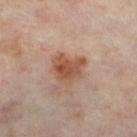Case summary:
– follow-up — imaged on a skin check; not biopsied
– site — the leg
– imaging modality — ~15 mm tile from a whole-body skin photo
– size — about 3.5 mm
– automated lesion analysis — a footprint of about 9 mm², an eccentricity of roughly 0.55, and two-axis asymmetry of about 0.35; an average lesion color of about L≈50 a*≈22 b*≈30 (CIELAB), a lesion–skin lightness drop of about 11, and a lesion-to-skin contrast of about 9 (normalized; higher = more distinct); a border-irregularity index near 3.5/10, a within-lesion color-variation index near 4/10, and a peripheral color-asymmetry measure near 1.5; a nevus-likeness score of about 95/100 and a lesion-detection confidence of about 100/100
– subject — female, about 55 years old
– tile lighting — cross-polarized illumination